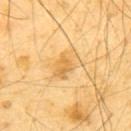This lesion was catalogued during total-body skin photography and was not selected for biopsy. Automated tile analysis of the lesion measured a border-irregularity index near 3/10 and peripheral color asymmetry of about 1.5. Located on the upper back. A 15 mm crop from a total-body photograph taken for skin-cancer surveillance. A male patient, in their mid-60s. The lesion's longest dimension is about 5 mm.